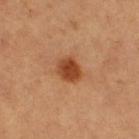biopsy status: no biopsy performed (imaged during a skin exam) | body site: the left leg | automated lesion analysis: a footprint of about 7 mm², an outline eccentricity of about 0.55 (0 = round, 1 = elongated), and a symmetry-axis asymmetry near 0.2; a normalized lesion–skin contrast near 9.5; a border-irregularity index near 1.5/10, a within-lesion color-variation index near 3.5/10, and a peripheral color-asymmetry measure near 1 | lighting: cross-polarized | image: 15 mm crop, total-body photography | patient: female, in their mid-40s.